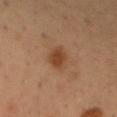No biopsy was performed on this lesion — it was imaged during a full skin examination and was not determined to be concerning. The subject is a male roughly 55 years of age. On the chest. A lesion tile, about 15 mm wide, cut from a 3D total-body photograph.